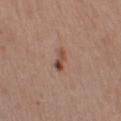This lesion was catalogued during total-body skin photography and was not selected for biopsy.
A male subject, aged 53 to 57.
On the left upper arm.
The lesion's longest dimension is about 3 mm.
The tile uses white-light illumination.
Cropped from a total-body skin-imaging series; the visible field is about 15 mm.
An algorithmic analysis of the crop reported an area of roughly 3.5 mm², an outline eccentricity of about 0.9 (0 = round, 1 = elongated), and two-axis asymmetry of about 0.35. The analysis additionally found a mean CIELAB color near L≈48 a*≈20 b*≈28, a lesion–skin lightness drop of about 11, and a normalized lesion–skin contrast near 8.5. The analysis additionally found an automated nevus-likeness rating near 75 out of 100 and lesion-presence confidence of about 100/100.